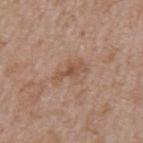follow-up=no biopsy performed (imaged during a skin exam); acquisition=total-body-photography crop, ~15 mm field of view; image-analysis metrics=a border-irregularity rating of about 4.5/10, a within-lesion color-variation index near 2.5/10, and a peripheral color-asymmetry measure near 0.5; subject=male, approximately 65 years of age; size=about 3.5 mm; body site=the mid back.